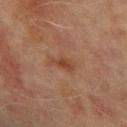This lesion was catalogued during total-body skin photography and was not selected for biopsy. From the left upper arm. A roughly 15 mm field-of-view crop from a total-body skin photograph. A male patient, aged 68 to 72. Captured under cross-polarized illumination.